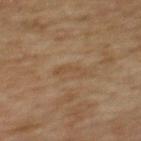Q: Was a biopsy performed?
A: catalogued during a skin exam; not biopsied
Q: Automated lesion metrics?
A: an area of roughly 3 mm² and a symmetry-axis asymmetry near 0.5
Q: Lesion location?
A: the back
Q: Who is the patient?
A: female, aged 58–62
Q: How was this image acquired?
A: ~15 mm tile from a whole-body skin photo
Q: What is the lesion's diameter?
A: ≈3.5 mm